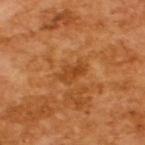Clinical impression:
This lesion was catalogued during total-body skin photography and was not selected for biopsy.
Context:
Cropped from a total-body skin-imaging series; the visible field is about 15 mm. Captured under cross-polarized illumination. The patient is a male in their mid-60s. An algorithmic analysis of the crop reported border irregularity of about 4 on a 0–10 scale, a within-lesion color-variation index near 3/10, and radial color variation of about 1. The recorded lesion diameter is about 3 mm.